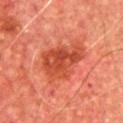Impression:
Recorded during total-body skin imaging; not selected for excision or biopsy.
Context:
Longest diameter approximately 6 mm. Cropped from a total-body skin-imaging series; the visible field is about 15 mm. The subject is a male aged approximately 65. The lesion is located on the chest. Imaged with cross-polarized lighting.